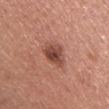Assessment:
Part of a total-body skin-imaging series; this lesion was reviewed on a skin check and was not flagged for biopsy.
Background:
A region of skin cropped from a whole-body photographic capture, roughly 15 mm wide. A male patient, aged 43–47. Automated tile analysis of the lesion measured a border-irregularity rating of about 2/10, a within-lesion color-variation index near 4.5/10, and radial color variation of about 1.5. The lesion is on the chest.